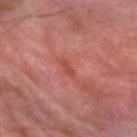Q: Was a biopsy performed?
A: no biopsy performed (imaged during a skin exam)
Q: Lesion size?
A: ≈3 mm
Q: What are the patient's age and sex?
A: male, aged 68–72
Q: What kind of image is this?
A: ~15 mm crop, total-body skin-cancer survey
Q: Where on the body is the lesion?
A: the left forearm